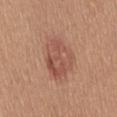Recorded during total-body skin imaging; not selected for excision or biopsy. A 15 mm close-up extracted from a 3D total-body photography capture. The lesion's longest dimension is about 5.5 mm. The total-body-photography lesion software estimated an average lesion color of about L≈53 a*≈23 b*≈28 (CIELAB) and roughly 9 lightness units darker than nearby skin. And it measured a border-irregularity index near 2/10, a within-lesion color-variation index near 5/10, and a peripheral color-asymmetry measure near 2. From the front of the torso. A female subject, about 55 years old. The tile uses white-light illumination.